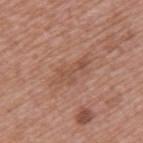<case>
<biopsy_status>not biopsied; imaged during a skin examination</biopsy_status>
<site>left upper arm</site>
<lesion_size>
  <long_diameter_mm_approx>5.0</long_diameter_mm_approx>
</lesion_size>
<image>
  <source>total-body photography crop</source>
  <field_of_view_mm>15</field_of_view_mm>
</image>
<patient>
  <sex>female</sex>
  <age_approx>45</age_approx>
</patient>
</case>